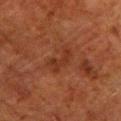Imaged during a routine full-body skin examination; the lesion was not biopsied and no histopathology is available. Captured under cross-polarized illumination. A roughly 15 mm field-of-view crop from a total-body skin photograph. Longest diameter approximately 3.5 mm. The patient is a male about 80 years old. On the left lower leg.